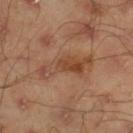Recorded during total-body skin imaging; not selected for excision or biopsy. A male patient roughly 45 years of age. Captured under cross-polarized illumination. This image is a 15 mm lesion crop taken from a total-body photograph. The lesion is on the right lower leg. The lesion's longest dimension is about 6.5 mm.